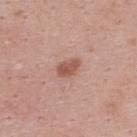Background:
Cropped from a total-body skin-imaging series; the visible field is about 15 mm. The patient is a male roughly 25 years of age. The total-body-photography lesion software estimated a footprint of about 4 mm² and an eccentricity of roughly 0.8. On the upper back. Captured under white-light illumination.